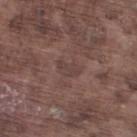Assessment: Captured during whole-body skin photography for melanoma surveillance; the lesion was not biopsied. Clinical summary: This image is a 15 mm lesion crop taken from a total-body photograph. The tile uses white-light illumination. The lesion is located on the right lower leg. The recorded lesion diameter is about 3 mm. The patient is a male aged approximately 75.